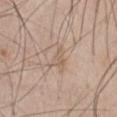Part of a total-body skin-imaging series; this lesion was reviewed on a skin check and was not flagged for biopsy.
A region of skin cropped from a whole-body photographic capture, roughly 15 mm wide.
Located on the abdomen.
A male patient, aged approximately 60.